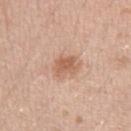| feature | finding |
|---|---|
| follow-up | total-body-photography surveillance lesion; no biopsy |
| automated metrics | an outline eccentricity of about 0.6 (0 = round, 1 = elongated) and two-axis asymmetry of about 0.25; about 10 CIELAB-L* units darker than the surrounding skin and a normalized border contrast of about 7; a border-irregularity index near 2.5/10, internal color variation of about 2 on a 0–10 scale, and radial color variation of about 0.5; an automated nevus-likeness rating near 40 out of 100 and lesion-presence confidence of about 100/100 |
| illumination | white-light illumination |
| lesion size | about 3 mm |
| subject | male, approximately 30 years of age |
| imaging modality | 15 mm crop, total-body photography |
| location | the left upper arm |